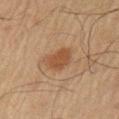Captured during whole-body skin photography for melanoma surveillance; the lesion was not biopsied.